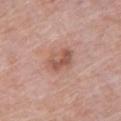Q: Was a biopsy performed?
A: catalogued during a skin exam; not biopsied
Q: How was the tile lit?
A: white-light illumination
Q: Lesion size?
A: ≈3.5 mm
Q: How was this image acquired?
A: ~15 mm crop, total-body skin-cancer survey
Q: What is the anatomic site?
A: the chest
Q: Who is the patient?
A: male, in their 80s